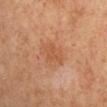Part of a total-body skin-imaging series; this lesion was reviewed on a skin check and was not flagged for biopsy.